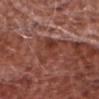Clinical impression: Recorded during total-body skin imaging; not selected for excision or biopsy. Context: A male patient roughly 75 years of age. About 4 mm across. Located on the head or neck. This is a white-light tile. A close-up tile cropped from a whole-body skin photograph, about 15 mm across.